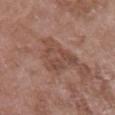<tbp_lesion>
  <biopsy_status>not biopsied; imaged during a skin examination</biopsy_status>
  <site>left upper arm</site>
  <patient>
    <sex>female</sex>
    <age_approx>70</age_approx>
  </patient>
  <image>
    <source>total-body photography crop</source>
    <field_of_view_mm>15</field_of_view_mm>
  </image>
</tbp_lesion>